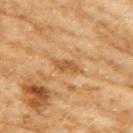The lesion is located on the right upper arm.
The subject is a female about 60 years old.
A 15 mm close-up tile from a total-body photography series done for melanoma screening.